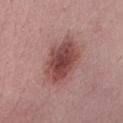This lesion was catalogued during total-body skin photography and was not selected for biopsy.
A female subject, in their 50s.
A 15 mm crop from a total-body photograph taken for skin-cancer surveillance.
The lesion is located on the abdomen.